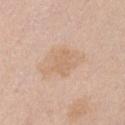Q: Was a biopsy performed?
A: no biopsy performed (imaged during a skin exam)
Q: How was this image acquired?
A: ~15 mm crop, total-body skin-cancer survey
Q: What is the lesion's diameter?
A: ~3.5 mm (longest diameter)
Q: Where on the body is the lesion?
A: the abdomen
Q: Illumination type?
A: white-light illumination
Q: Patient demographics?
A: female, approximately 65 years of age